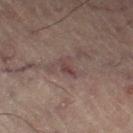biopsy status — total-body-photography surveillance lesion; no biopsy | lighting — cross-polarized illumination | anatomic site — the left thigh | diameter — about 2.5 mm | imaging modality — ~15 mm crop, total-body skin-cancer survey | subject — male, aged approximately 60 | image-analysis metrics — a mean CIELAB color near L≈36 a*≈16 b*≈16, about 6 CIELAB-L* units darker than the surrounding skin, and a normalized lesion–skin contrast near 6; border irregularity of about 5 on a 0–10 scale, a within-lesion color-variation index near 1/10, and peripheral color asymmetry of about 0.